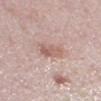Part of a total-body skin-imaging series; this lesion was reviewed on a skin check and was not flagged for biopsy. Cropped from a whole-body photographic skin survey; the tile spans about 15 mm. On the left lower leg. A female subject, aged 38 to 42. Captured under white-light illumination.